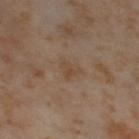Acquisition and patient details:
Located on the leg. The recorded lesion diameter is about 3 mm. A female subject, in their mid-50s. An algorithmic analysis of the crop reported a classifier nevus-likeness of about 0/100 and a lesion-detection confidence of about 100/100. A 15 mm close-up extracted from a 3D total-body photography capture. Imaged with cross-polarized lighting.